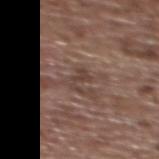Q: Was a biopsy performed?
A: total-body-photography surveillance lesion; no biopsy
Q: Illumination type?
A: white-light illumination
Q: Where on the body is the lesion?
A: the upper back
Q: What kind of image is this?
A: ~15 mm crop, total-body skin-cancer survey
Q: How large is the lesion?
A: about 2.5 mm
Q: What are the patient's age and sex?
A: female, roughly 65 years of age